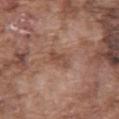Q: How large is the lesion?
A: ≈3 mm
Q: What is the imaging modality?
A: 15 mm crop, total-body photography
Q: What are the patient's age and sex?
A: male, in their mid- to late 70s
Q: What is the anatomic site?
A: the front of the torso
Q: How was the tile lit?
A: white-light